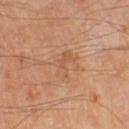Impression:
Imaged during a routine full-body skin examination; the lesion was not biopsied and no histopathology is available.
Background:
The tile uses cross-polarized illumination. From the left thigh. A male subject about 65 years old. Approximately 4 mm at its widest. A region of skin cropped from a whole-body photographic capture, roughly 15 mm wide.